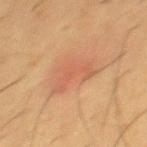Findings:
• subject · male, aged around 55
• TBP lesion metrics · a lesion area of about 11 mm², an eccentricity of roughly 0.8, and two-axis asymmetry of about 0.45; a lesion color around L≈51 a*≈23 b*≈31 in CIELAB, a lesion–skin lightness drop of about 6, and a normalized border contrast of about 4.5; border irregularity of about 5.5 on a 0–10 scale, a color-variation rating of about 2.5/10, and a peripheral color-asymmetry measure near 1; a nevus-likeness score of about 5/100 and a detector confidence of about 100 out of 100 that the crop contains a lesion
• imaging modality · total-body-photography crop, ~15 mm field of view
• location · the chest
• illumination · cross-polarized